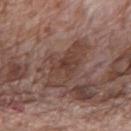* biopsy status · imaged on a skin check; not biopsied
* patient · male, aged 68 to 72
* acquisition · ~15 mm tile from a whole-body skin photo
* site · the back